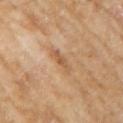No biopsy was performed on this lesion — it was imaged during a full skin examination and was not determined to be concerning. On the arm. A 15 mm close-up extracted from a 3D total-body photography capture. A female subject, approximately 60 years of age.